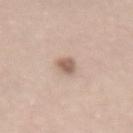Impression: This lesion was catalogued during total-body skin photography and was not selected for biopsy. Clinical summary: The lesion is located on the mid back. The tile uses white-light illumination. The patient is a male aged around 60. Approximately 2.5 mm at its widest. Automated tile analysis of the lesion measured an outline eccentricity of about 0.75 (0 = round, 1 = elongated). It also reported a color-variation rating of about 2/10 and radial color variation of about 0.5. A 15 mm close-up tile from a total-body photography series done for melanoma screening.